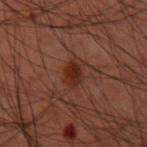Assessment:
The lesion was tiled from a total-body skin photograph and was not biopsied.
Context:
Located on the right forearm. The lesion-visualizer software estimated a lesion area of about 6 mm² and an eccentricity of roughly 0.85. The software also gave a border-irregularity rating of about 2.5/10, a within-lesion color-variation index near 2/10, and radial color variation of about 0.5. The lesion's longest dimension is about 4 mm. This is a cross-polarized tile. The subject is a male about 60 years old. A 15 mm crop from a total-body photograph taken for skin-cancer surveillance.